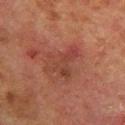Clinical impression:
No biopsy was performed on this lesion — it was imaged during a full skin examination and was not determined to be concerning.
Clinical summary:
The lesion is located on the left lower leg. A male subject, aged 78–82. Longest diameter approximately 4.5 mm. A region of skin cropped from a whole-body photographic capture, roughly 15 mm wide.